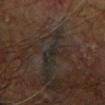| field | value |
|---|---|
| patient | male, aged 68 to 72 |
| acquisition | total-body-photography crop, ~15 mm field of view |
| illumination | cross-polarized |
| anatomic site | the left forearm |
| size | about 1.5 mm |